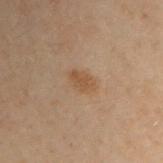Impression: Imaged during a routine full-body skin examination; the lesion was not biopsied and no histopathology is available. Background: The tile uses cross-polarized illumination. Longest diameter approximately 3 mm. A male subject, aged approximately 50. A 15 mm crop from a total-body photograph taken for skin-cancer surveillance. Automated tile analysis of the lesion measured an eccentricity of roughly 0.75 and a shape-asymmetry score of about 0.25 (0 = symmetric). The lesion is located on the left arm.